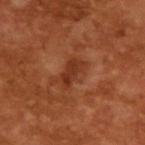Assessment:
The lesion was tiled from a total-body skin photograph and was not biopsied.
Background:
The lesion's longest dimension is about 3.5 mm. The lesion-visualizer software estimated an eccentricity of roughly 0.85 and a symmetry-axis asymmetry near 0.3. And it measured a mean CIELAB color near L≈34 a*≈26 b*≈33, about 8 CIELAB-L* units darker than the surrounding skin, and a normalized border contrast of about 7. The software also gave a border-irregularity rating of about 3.5/10, internal color variation of about 2.5 on a 0–10 scale, and peripheral color asymmetry of about 1. The software also gave a nevus-likeness score of about 5/100. A male subject aged around 65. A 15 mm crop from a total-body photograph taken for skin-cancer surveillance. The tile uses cross-polarized illumination.